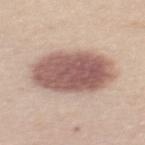Captured under white-light illumination. The patient is a female about 55 years old. A 15 mm close-up tile from a total-body photography series done for melanoma screening. Located on the back.